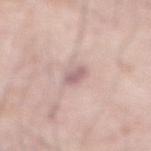<case>
<biopsy_status>not biopsied; imaged during a skin examination</biopsy_status>
<lesion_size>
  <long_diameter_mm_approx>2.5</long_diameter_mm_approx>
</lesion_size>
<lighting>white-light</lighting>
<site>abdomen</site>
<image>
  <source>total-body photography crop</source>
  <field_of_view_mm>15</field_of_view_mm>
</image>
<patient>
  <sex>male</sex>
  <age_approx>60</age_approx>
</patient>
</case>